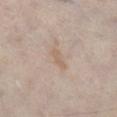workup: imaged on a skin check; not biopsied | tile lighting: white-light | body site: the right lower leg | acquisition: 15 mm crop, total-body photography | subject: male, roughly 60 years of age.